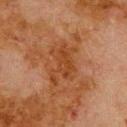A male patient, aged approximately 80. Captured under cross-polarized illumination. Measured at roughly 5.5 mm in maximum diameter. On the upper back. A roughly 15 mm field-of-view crop from a total-body skin photograph. The lesion-visualizer software estimated an area of roughly 12 mm², a shape eccentricity near 0.8, and two-axis asymmetry of about 0.5. And it measured a border-irregularity index near 7.5/10, a color-variation rating of about 2.5/10, and a peripheral color-asymmetry measure near 1. It also reported a nevus-likeness score of about 0/100 and lesion-presence confidence of about 100/100.